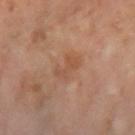The lesion was photographed on a routine skin check and not biopsied; there is no pathology result. A female patient, in their mid- to late 60s. Captured under cross-polarized illumination. Cropped from a whole-body photographic skin survey; the tile spans about 15 mm. Approximately 3 mm at its widest. Located on the left forearm.